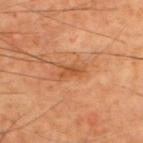notes: no biopsy performed (imaged during a skin exam); illumination: cross-polarized; subject: male, in their 60s; location: the upper back; lesion diameter: about 3 mm; image: 15 mm crop, total-body photography; image-analysis metrics: border irregularity of about 4 on a 0–10 scale, a within-lesion color-variation index near 2/10, and a peripheral color-asymmetry measure near 1.